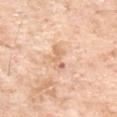Clinical impression: This lesion was catalogued during total-body skin photography and was not selected for biopsy. Image and clinical context: The lesion's longest dimension is about 3.5 mm. A 15 mm close-up extracted from a 3D total-body photography capture. Imaged with white-light lighting. Located on the front of the torso. The subject is a male approximately 70 years of age.